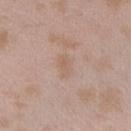Impression:
Captured during whole-body skin photography for melanoma surveillance; the lesion was not biopsied.
Image and clinical context:
The tile uses white-light illumination. A female subject aged 23–27. An algorithmic analysis of the crop reported a footprint of about 4 mm², an outline eccentricity of about 0.8 (0 = round, 1 = elongated), and two-axis asymmetry of about 0.25. And it measured border irregularity of about 2.5 on a 0–10 scale, a color-variation rating of about 2/10, and radial color variation of about 0.5. Cropped from a total-body skin-imaging series; the visible field is about 15 mm. On the left forearm.